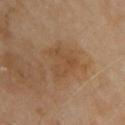<case>
<biopsy_status>not biopsied; imaged during a skin examination</biopsy_status>
<patient>
  <sex>male</sex>
  <age_approx>70</age_approx>
</patient>
<image>
  <source>total-body photography crop</source>
  <field_of_view_mm>15</field_of_view_mm>
</image>
<site>chest</site>
</case>